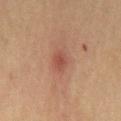| feature | finding |
|---|---|
| notes | no biopsy performed (imaged during a skin exam) |
| imaging modality | ~15 mm tile from a whole-body skin photo |
| lesion diameter | ~2.5 mm (longest diameter) |
| automated lesion analysis | a lesion color around L≈43 a*≈23 b*≈25 in CIELAB and a lesion-to-skin contrast of about 6.5 (normalized; higher = more distinct); border irregularity of about 1.5 on a 0–10 scale and internal color variation of about 1.5 on a 0–10 scale |
| subject | female, aged around 70 |
| illumination | cross-polarized |
| anatomic site | the mid back |